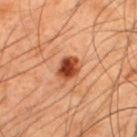A male subject aged approximately 45.
This is a cross-polarized tile.
The lesion is located on the upper back.
A roughly 15 mm field-of-view crop from a total-body skin photograph.
The lesion's longest dimension is about 3 mm.
Automated image analysis of the tile measured an area of roughly 5.5 mm² and two-axis asymmetry of about 0.2. The software also gave a border-irregularity index near 2/10 and internal color variation of about 5 on a 0–10 scale. The software also gave a nevus-likeness score of about 100/100 and lesion-presence confidence of about 100/100.